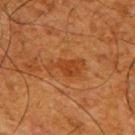Case summary:
– notes: total-body-photography surveillance lesion; no biopsy
– illumination: cross-polarized illumination
– patient: male, in their mid- to late 60s
– diameter: ≈4.5 mm
– site: the upper back
– image source: 15 mm crop, total-body photography